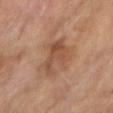Q: Was a biopsy performed?
A: imaged on a skin check; not biopsied
Q: What did automated image analysis measure?
A: a mean CIELAB color near L≈49 a*≈21 b*≈31 and roughly 8 lightness units darker than nearby skin; a classifier nevus-likeness of about 10/100 and a detector confidence of about 100 out of 100 that the crop contains a lesion
Q: What is the anatomic site?
A: the right forearm
Q: What is the imaging modality?
A: 15 mm crop, total-body photography
Q: Lesion size?
A: about 5 mm
Q: Patient demographics?
A: female, aged 58 to 62